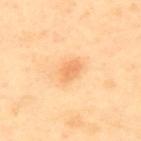This lesion was catalogued during total-body skin photography and was not selected for biopsy. This image is a 15 mm lesion crop taken from a total-body photograph. Captured under cross-polarized illumination. From the upper back. The total-body-photography lesion software estimated an eccentricity of roughly 0.75 and a symmetry-axis asymmetry near 0.25. The analysis additionally found a mean CIELAB color near L≈75 a*≈26 b*≈46 and a lesion-to-skin contrast of about 5.5 (normalized; higher = more distinct). The analysis additionally found border irregularity of about 2 on a 0–10 scale and internal color variation of about 2 on a 0–10 scale. Approximately 3 mm at its widest. The subject is a female in their 40s.